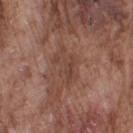This lesion was catalogued during total-body skin photography and was not selected for biopsy. A male patient, aged 73 to 77. The lesion is on the right upper arm. A region of skin cropped from a whole-body photographic capture, roughly 15 mm wide. The recorded lesion diameter is about 4.5 mm.